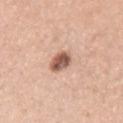| feature | finding |
|---|---|
| notes | no biopsy performed (imaged during a skin exam) |
| TBP lesion metrics | a shape eccentricity near 0.5 and a shape-asymmetry score of about 0.2 (0 = symmetric); a border-irregularity index near 1.5/10, a within-lesion color-variation index near 5.5/10, and a peripheral color-asymmetry measure near 1.5; a nevus-likeness score of about 70/100 and a lesion-detection confidence of about 100/100 |
| illumination | white-light |
| lesion size | about 3 mm |
| subject | female, aged 43 to 47 |
| body site | the arm |
| imaging modality | total-body-photography crop, ~15 mm field of view |